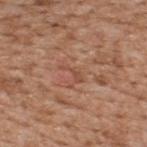The lesion was tiled from a total-body skin photograph and was not biopsied. The lesion is on the upper back. The patient is a male aged 63–67. Cropped from a total-body skin-imaging series; the visible field is about 15 mm.